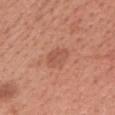{
  "biopsy_status": "not biopsied; imaged during a skin examination",
  "automated_metrics": {
    "color_variation_0_10": 2.5,
    "peripheral_color_asymmetry": 1.0
  },
  "patient": {
    "sex": "female",
    "age_approx": 50
  },
  "site": "head or neck",
  "lesion_size": {
    "long_diameter_mm_approx": 3.5
  },
  "image": {
    "source": "total-body photography crop",
    "field_of_view_mm": 15
  },
  "lighting": "white-light"
}